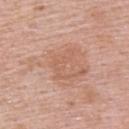Assessment: Part of a total-body skin-imaging series; this lesion was reviewed on a skin check and was not flagged for biopsy. Clinical summary: A region of skin cropped from a whole-body photographic capture, roughly 15 mm wide. The recorded lesion diameter is about 7 mm. A male patient approximately 55 years of age. Located on the upper back.